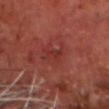Imaged during a routine full-body skin examination; the lesion was not biopsied and no histopathology is available. This image is a 15 mm lesion crop taken from a total-body photograph. An algorithmic analysis of the crop reported an area of roughly 3 mm², an outline eccentricity of about 0.85 (0 = round, 1 = elongated), and a shape-asymmetry score of about 0.45 (0 = symmetric). The analysis additionally found about 5 CIELAB-L* units darker than the surrounding skin and a normalized lesion–skin contrast near 5. A male patient, about 70 years old. The recorded lesion diameter is about 2.5 mm. The lesion is located on the head or neck.